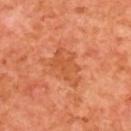Q: Was a biopsy performed?
A: total-body-photography surveillance lesion; no biopsy
Q: How was this image acquired?
A: 15 mm crop, total-body photography
Q: How was the tile lit?
A: cross-polarized illumination
Q: Who is the patient?
A: female, in their 40s
Q: How large is the lesion?
A: about 5 mm
Q: Lesion location?
A: the back